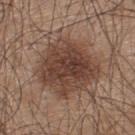{"biopsy_status": "not biopsied; imaged during a skin examination", "site": "upper back", "patient": {"sex": "male", "age_approx": 45}, "image": {"source": "total-body photography crop", "field_of_view_mm": 15}, "lesion_size": {"long_diameter_mm_approx": 7.0}}